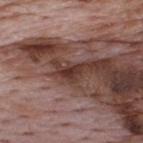{"site": "upper back", "lesion_size": {"long_diameter_mm_approx": 2.5}, "image": {"source": "total-body photography crop", "field_of_view_mm": 15}, "lighting": "white-light", "patient": {"sex": "male", "age_approx": 70}}